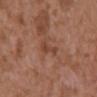Findings:
- diameter: about 3 mm
- patient: male, aged 73–77
- lighting: white-light illumination
- body site: the front of the torso
- acquisition: ~15 mm crop, total-body skin-cancer survey